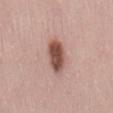Clinical impression: Recorded during total-body skin imaging; not selected for excision or biopsy. Acquisition and patient details: A 15 mm close-up extracted from a 3D total-body photography capture. Approximately 4.5 mm at its widest. Automated image analysis of the tile measured a lesion area of about 9 mm², an outline eccentricity of about 0.85 (0 = round, 1 = elongated), and two-axis asymmetry of about 0.15. It also reported about 16 CIELAB-L* units darker than the surrounding skin and a normalized lesion–skin contrast near 11. The software also gave lesion-presence confidence of about 100/100. The lesion is located on the mid back. A female subject, approximately 30 years of age. The tile uses white-light illumination.